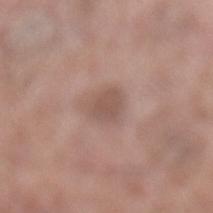The lesion was tiled from a total-body skin photograph and was not biopsied. A region of skin cropped from a whole-body photographic capture, roughly 15 mm wide. A female patient approximately 50 years of age. From the left lower leg. The tile uses white-light illumination.